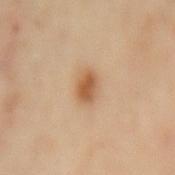Captured during whole-body skin photography for melanoma surveillance; the lesion was not biopsied. The lesion is on the mid back. The subject is a female approximately 60 years of age. Cropped from a total-body skin-imaging series; the visible field is about 15 mm.